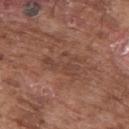Imaged during a routine full-body skin examination; the lesion was not biopsied and no histopathology is available. A male subject aged approximately 75. Automated tile analysis of the lesion measured roughly 6 lightness units darker than nearby skin and a normalized lesion–skin contrast near 5. And it measured a border-irregularity index near 8.5/10 and a peripheral color-asymmetry measure near 1. The analysis additionally found an automated nevus-likeness rating near 0 out of 100. Measured at roughly 4 mm in maximum diameter. The lesion is located on the right upper arm. Cropped from a total-body skin-imaging series; the visible field is about 15 mm.